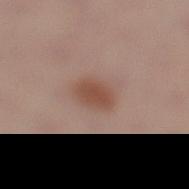- biopsy status · imaged on a skin check; not biopsied
- anatomic site · the left lower leg
- acquisition · ~15 mm tile from a whole-body skin photo
- TBP lesion metrics · a footprint of about 6.5 mm²; a mean CIELAB color near L≈49 a*≈20 b*≈27, roughly 9 lightness units darker than nearby skin, and a lesion-to-skin contrast of about 7.5 (normalized; higher = more distinct)
- subject · female, approximately 40 years of age
- illumination · white-light illumination